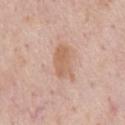| feature | finding |
|---|---|
| workup | imaged on a skin check; not biopsied |
| lighting | white-light |
| lesion diameter | ~4 mm (longest diameter) |
| anatomic site | the front of the torso |
| acquisition | ~15 mm crop, total-body skin-cancer survey |
| subject | male, about 60 years old |